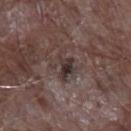{"biopsy_status": "not biopsied; imaged during a skin examination", "lesion_size": {"long_diameter_mm_approx": 4.0}, "patient": {"sex": "male", "age_approx": 65}, "site": "left forearm", "automated_metrics": {"area_mm2_approx": 6.0, "cielab_L": 34, "cielab_a": 13, "cielab_b": 15, "vs_skin_darker_L": 9.0, "vs_skin_contrast_norm": 8.5, "nevus_likeness_0_100": 0, "lesion_detection_confidence_0_100": 80}, "lighting": "white-light", "image": {"source": "total-body photography crop", "field_of_view_mm": 15}}